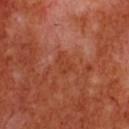  automated_metrics:
    cielab_L: 43
    cielab_a: 32
    cielab_b: 36
    vs_skin_darker_L: 5.0
    vs_skin_contrast_norm: 5.0
  lesion_size:
    long_diameter_mm_approx: 2.5
  site: chest
  image:
    source: total-body photography crop
    field_of_view_mm: 15
  lighting: cross-polarized
  patient:
    sex: male
    age_approx: 55